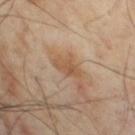follow-up: no biopsy performed (imaged during a skin exam) | subject: male, aged 68–72 | image source: ~15 mm crop, total-body skin-cancer survey | location: the chest.